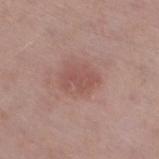A close-up tile cropped from a whole-body skin photograph, about 15 mm across. The subject is a male roughly 70 years of age. The recorded lesion diameter is about 3.5 mm. On the leg. Captured under white-light illumination.